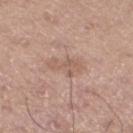Clinical impression:
No biopsy was performed on this lesion — it was imaged during a full skin examination and was not determined to be concerning.
Background:
This is a white-light tile. A male patient approximately 65 years of age. On the left lower leg. The recorded lesion diameter is about 3.5 mm. A close-up tile cropped from a whole-body skin photograph, about 15 mm across. Automated image analysis of the tile measured a nevus-likeness score of about 0/100.